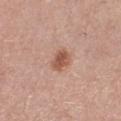workup = imaged on a skin check; not biopsied | location = the right lower leg | lighting = white-light illumination | subject = female, aged 53 to 57 | diameter = ~2.5 mm (longest diameter) | acquisition = ~15 mm tile from a whole-body skin photo.